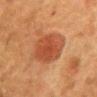<lesion>
<biopsy_status>not biopsied; imaged during a skin examination</biopsy_status>
<lesion_size>
  <long_diameter_mm_approx>5.0</long_diameter_mm_approx>
</lesion_size>
<patient>
  <sex>female</sex>
  <age_approx>50</age_approx>
</patient>
<site>chest</site>
<image>
  <source>total-body photography crop</source>
  <field_of_view_mm>15</field_of_view_mm>
</image>
<lighting>cross-polarized</lighting>
</lesion>